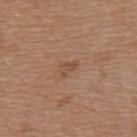This lesion was catalogued during total-body skin photography and was not selected for biopsy.
The lesion's longest dimension is about 2.5 mm.
From the upper back.
Automated image analysis of the tile measured a footprint of about 3 mm², an outline eccentricity of about 0.85 (0 = round, 1 = elongated), and two-axis asymmetry of about 0.45. The software also gave a border-irregularity index near 4.5/10, a color-variation rating of about 1.5/10, and radial color variation of about 0.5.
A female subject, aged approximately 55.
This image is a 15 mm lesion crop taken from a total-body photograph.